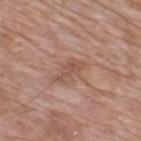<record>
  <biopsy_status>not biopsied; imaged during a skin examination</biopsy_status>
  <patient>
    <sex>male</sex>
    <age_approx>60</age_approx>
  </patient>
  <site>upper back</site>
  <image>
    <source>total-body photography crop</source>
    <field_of_view_mm>15</field_of_view_mm>
  </image>
</record>